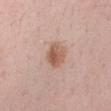| feature | finding |
|---|---|
| workup | no biopsy performed (imaged during a skin exam) |
| image source | 15 mm crop, total-body photography |
| patient | female, in their mid-30s |
| site | the right upper arm |